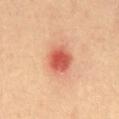No biopsy was performed on this lesion — it was imaged during a full skin examination and was not determined to be concerning.